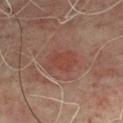follow-up: imaged on a skin check; not biopsied
image: ~15 mm tile from a whole-body skin photo
body site: the back
subject: male, about 70 years old
tile lighting: cross-polarized
diameter: ≈4 mm